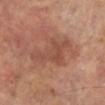The lesion was photographed on a routine skin check and not biopsied; there is no pathology result.
Approximately 6.5 mm at its widest.
From the left lower leg.
The total-body-photography lesion software estimated a mean CIELAB color near L≈47 a*≈23 b*≈28, roughly 8 lightness units darker than nearby skin, and a normalized border contrast of about 6. The software also gave border irregularity of about 7.5 on a 0–10 scale, internal color variation of about 2 on a 0–10 scale, and radial color variation of about 0.5.
The subject is a male approximately 70 years of age.
A region of skin cropped from a whole-body photographic capture, roughly 15 mm wide.
Captured under cross-polarized illumination.